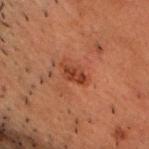| key | value |
|---|---|
| biopsy status | total-body-photography surveillance lesion; no biopsy |
| image-analysis metrics | a classifier nevus-likeness of about 15/100 and lesion-presence confidence of about 100/100 |
| acquisition | ~15 mm tile from a whole-body skin photo |
| location | the chest |
| tile lighting | cross-polarized illumination |
| lesion size | ~3 mm (longest diameter) |
| patient | male, about 50 years old |